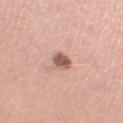The lesion was tiled from a total-body skin photograph and was not biopsied.